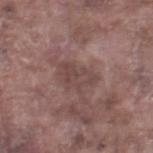The lesion was tiled from a total-body skin photograph and was not biopsied. The lesion is located on the right thigh. This is a white-light tile. A 15 mm close-up extracted from a 3D total-body photography capture. A male patient in their mid-70s. Measured at roughly 4.5 mm in maximum diameter. An algorithmic analysis of the crop reported an average lesion color of about L≈44 a*≈18 b*≈20 (CIELAB), a lesion–skin lightness drop of about 7, and a lesion-to-skin contrast of about 5.5 (normalized; higher = more distinct). The software also gave border irregularity of about 4 on a 0–10 scale, internal color variation of about 3 on a 0–10 scale, and peripheral color asymmetry of about 1. It also reported an automated nevus-likeness rating near 0 out of 100 and lesion-presence confidence of about 100/100.